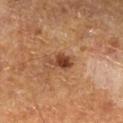The lesion was tiled from a total-body skin photograph and was not biopsied.
A 15 mm crop from a total-body photograph taken for skin-cancer surveillance.
A male subject aged around 60.
The total-body-photography lesion software estimated internal color variation of about 4 on a 0–10 scale and a peripheral color-asymmetry measure near 1.5. It also reported a classifier nevus-likeness of about 85/100 and a lesion-detection confidence of about 100/100.
Located on the left lower leg.
Approximately 3 mm at its widest.